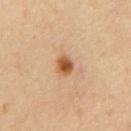Measured at roughly 2.5 mm in maximum diameter. Captured under cross-polarized illumination. On the chest. Cropped from a total-body skin-imaging series; the visible field is about 15 mm. Automated tile analysis of the lesion measured border irregularity of about 2 on a 0–10 scale, a within-lesion color-variation index near 4/10, and peripheral color asymmetry of about 1. It also reported a nevus-likeness score of about 100/100 and a lesion-detection confidence of about 100/100. A male subject aged around 60.